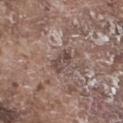Findings:
• workup: no biopsy performed (imaged during a skin exam)
• subject: male, aged 73–77
• lighting: white-light
• image source: ~15 mm crop, total-body skin-cancer survey
• site: the right thigh